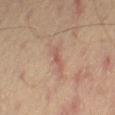* follow-up — imaged on a skin check; not biopsied
* illumination — cross-polarized
* patient — male, aged approximately 65
* site — the right thigh
* image — ~15 mm tile from a whole-body skin photo